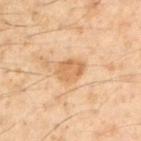workup: no biopsy performed (imaged during a skin exam) | location: the left upper arm | size: ≈3.5 mm | image: ~15 mm tile from a whole-body skin photo | patient: male, about 50 years old | lighting: cross-polarized illumination.